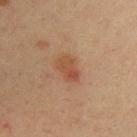Q: Was a biopsy performed?
A: total-body-photography surveillance lesion; no biopsy
Q: What is the imaging modality?
A: ~15 mm crop, total-body skin-cancer survey
Q: Who is the patient?
A: male, roughly 35 years of age
Q: Illumination type?
A: cross-polarized
Q: Where on the body is the lesion?
A: the chest
Q: Lesion size?
A: about 3 mm